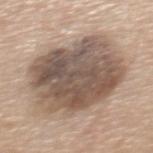Q: Was a biopsy performed?
A: total-body-photography surveillance lesion; no biopsy
Q: What kind of image is this?
A: ~15 mm crop, total-body skin-cancer survey
Q: What is the anatomic site?
A: the upper back
Q: Who is the patient?
A: female, aged around 65
Q: How large is the lesion?
A: ~9.5 mm (longest diameter)
Q: How was the tile lit?
A: white-light
Q: What did automated image analysis measure?
A: a border-irregularity index near 2.5/10, internal color variation of about 6.5 on a 0–10 scale, and radial color variation of about 2